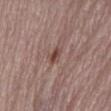– anatomic site: the left lower leg
– patient: female, aged 63 to 67
– imaging modality: ~15 mm crop, total-body skin-cancer survey
– lesion size: about 2.5 mm
– TBP lesion metrics: a shape-asymmetry score of about 0.3 (0 = symmetric); a border-irregularity rating of about 2.5/10 and a within-lesion color-variation index near 1.5/10; a classifier nevus-likeness of about 5/100 and a lesion-detection confidence of about 100/100
– tile lighting: white-light